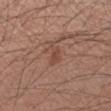The patient is a male approximately 35 years of age.
A lesion tile, about 15 mm wide, cut from a 3D total-body photograph.
This is a white-light tile.
The lesion-visualizer software estimated a footprint of about 4 mm², an outline eccentricity of about 0.75 (0 = round, 1 = elongated), and a symmetry-axis asymmetry near 0.5. And it measured peripheral color asymmetry of about 0.5. And it measured a classifier nevus-likeness of about 30/100.
Located on the left forearm.